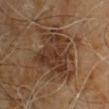<lesion>
  <biopsy_status>not biopsied; imaged during a skin examination</biopsy_status>
  <patient>
    <sex>male</sex>
    <age_approx>60</age_approx>
  </patient>
  <automated_metrics>
    <vs_skin_darker_L>9.0</vs_skin_darker_L>
    <vs_skin_contrast_norm>8.5</vs_skin_contrast_norm>
    <border_irregularity_0_10>7.0</border_irregularity_0_10>
    <color_variation_0_10>4.0</color_variation_0_10>
    <nevus_likeness_0_100>0</nevus_likeness_0_100>
  </automated_metrics>
  <image>
    <source>total-body photography crop</source>
    <field_of_view_mm>15</field_of_view_mm>
  </image>
  <lighting>cross-polarized</lighting>
  <site>upper back</site>
  <lesion_size>
    <long_diameter_mm_approx>7.5</long_diameter_mm_approx>
  </lesion_size>
</lesion>